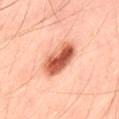Assessment: Imaged during a routine full-body skin examination; the lesion was not biopsied and no histopathology is available. Context: The patient is a male about 45 years old. The lesion is on the leg. This image is a 15 mm lesion crop taken from a total-body photograph. Measured at roughly 5 mm in maximum diameter.